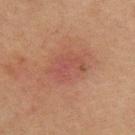| field | value |
|---|---|
| biopsy status | no biopsy performed (imaged during a skin exam) |
| subject | male, aged approximately 60 |
| automated lesion analysis | a footprint of about 10 mm² and a shape-asymmetry score of about 0.25 (0 = symmetric); a lesion–skin lightness drop of about 6 and a normalized lesion–skin contrast near 5; a border-irregularity index near 4/10 and a peripheral color-asymmetry measure near 1 |
| site | the upper back |
| image | total-body-photography crop, ~15 mm field of view |